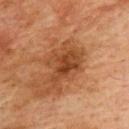Impression: The lesion was tiled from a total-body skin photograph and was not biopsied. Image and clinical context: Captured under cross-polarized illumination. The lesion is located on the upper back. A lesion tile, about 15 mm wide, cut from a 3D total-body photograph. A male patient, aged 73–77. Longest diameter approximately 5.5 mm.